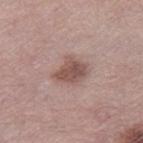<tbp_lesion>
<biopsy_status>not biopsied; imaged during a skin examination</biopsy_status>
<patient>
  <sex>female</sex>
  <age_approx>65</age_approx>
</patient>
<lesion_size>
  <long_diameter_mm_approx>3.5</long_diameter_mm_approx>
</lesion_size>
<image>
  <source>total-body photography crop</source>
  <field_of_view_mm>15</field_of_view_mm>
</image>
<site>right thigh</site>
</tbp_lesion>